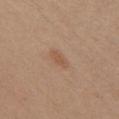biopsy status: catalogued during a skin exam; not biopsied | subject: female, aged 28 to 32 | image source: 15 mm crop, total-body photography | body site: the chest.